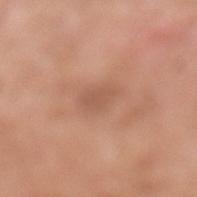Notes:
- notes — imaged on a skin check; not biopsied
- lesion size — ~3 mm (longest diameter)
- automated lesion analysis — a footprint of about 5.5 mm², an outline eccentricity of about 0.6 (0 = round, 1 = elongated), and a shape-asymmetry score of about 0.3 (0 = symmetric); an average lesion color of about L≈56 a*≈22 b*≈31 (CIELAB), a lesion–skin lightness drop of about 7, and a normalized border contrast of about 4.5; peripheral color asymmetry of about 0.5
- anatomic site — the leg
- image — ~15 mm crop, total-body skin-cancer survey
- lighting — white-light illumination
- patient — male, aged approximately 80